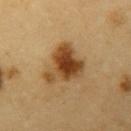Clinical impression:
Captured during whole-body skin photography for melanoma surveillance; the lesion was not biopsied.
Clinical summary:
Automated tile analysis of the lesion measured lesion-presence confidence of about 100/100. This image is a 15 mm lesion crop taken from a total-body photograph. Located on the left upper arm. The recorded lesion diameter is about 5 mm. A male patient, aged approximately 60.